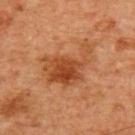• notes — no biopsy performed (imaged during a skin exam)
• body site — the chest
• acquisition — ~15 mm crop, total-body skin-cancer survey
• lesion diameter — ≈6.5 mm
• image-analysis metrics — a lesion area of about 18 mm², an eccentricity of roughly 0.8, and a shape-asymmetry score of about 0.5 (0 = symmetric)
• patient — male, about 60 years old